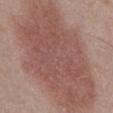image = ~15 mm tile from a whole-body skin photo; tile lighting = white-light; location = the abdomen; size = ≈15.5 mm; subject = male, in their mid-50s.